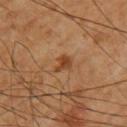Captured during whole-body skin photography for melanoma surveillance; the lesion was not biopsied. The lesion is located on the right upper arm. A 15 mm close-up extracted from a 3D total-body photography capture. A male subject roughly 60 years of age.